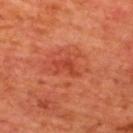workup: total-body-photography surveillance lesion; no biopsy
subject: male, in their mid- to late 60s
lighting: cross-polarized
lesion diameter: ~3.5 mm (longest diameter)
body site: the upper back
image source: 15 mm crop, total-body photography